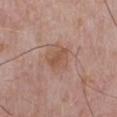Assessment: Recorded during total-body skin imaging; not selected for excision or biopsy. Background: Longest diameter approximately 3 mm. A roughly 15 mm field-of-view crop from a total-body skin photograph. An algorithmic analysis of the crop reported border irregularity of about 3 on a 0–10 scale, internal color variation of about 2.5 on a 0–10 scale, and radial color variation of about 1. Captured under white-light illumination. A male subject aged 63–67. The lesion is on the chest.